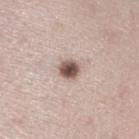Q: Was a biopsy performed?
A: imaged on a skin check; not biopsied
Q: Where on the body is the lesion?
A: the left lower leg
Q: How was this image acquired?
A: total-body-photography crop, ~15 mm field of view
Q: What are the patient's age and sex?
A: male, aged 58 to 62
Q: How large is the lesion?
A: about 2.5 mm